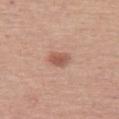Q: Is there a histopathology result?
A: imaged on a skin check; not biopsied
Q: What is the anatomic site?
A: the left thigh
Q: What did automated image analysis measure?
A: border irregularity of about 3 on a 0–10 scale and a color-variation rating of about 2/10; a nevus-likeness score of about 90/100 and lesion-presence confidence of about 100/100
Q: What kind of image is this?
A: total-body-photography crop, ~15 mm field of view
Q: What lighting was used for the tile?
A: white-light illumination
Q: Who is the patient?
A: female, roughly 50 years of age
Q: Lesion size?
A: ≈2.5 mm